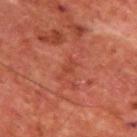Part of a total-body skin-imaging series; this lesion was reviewed on a skin check and was not flagged for biopsy.
The total-body-photography lesion software estimated a lesion color around L≈40 a*≈30 b*≈31 in CIELAB and a lesion–skin lightness drop of about 5. The analysis additionally found internal color variation of about 0 on a 0–10 scale and peripheral color asymmetry of about 0. And it measured a nevus-likeness score of about 0/100 and a detector confidence of about 100 out of 100 that the crop contains a lesion.
Captured under cross-polarized illumination.
On the upper back.
The patient is a male aged 63 to 67.
A 15 mm close-up tile from a total-body photography series done for melanoma screening.